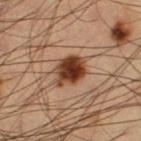anatomic site: the left thigh | image source: total-body-photography crop, ~15 mm field of view | subject: male, aged 58–62.